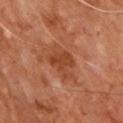notes = no biopsy performed (imaged during a skin exam); lighting = cross-polarized illumination; imaging modality = ~15 mm tile from a whole-body skin photo; TBP lesion metrics = a lesion color around L≈43 a*≈27 b*≈35 in CIELAB and roughly 8 lightness units darker than nearby skin; patient = male, roughly 65 years of age.